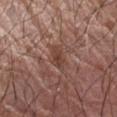A close-up tile cropped from a whole-body skin photograph, about 15 mm across.
The lesion's longest dimension is about 3 mm.
A male patient, in their 70s.
The lesion-visualizer software estimated a footprint of about 3.5 mm², an outline eccentricity of about 0.8 (0 = round, 1 = elongated), and two-axis asymmetry of about 0.3. The software also gave an average lesion color of about L≈41 a*≈21 b*≈24 (CIELAB), about 8 CIELAB-L* units darker than the surrounding skin, and a lesion-to-skin contrast of about 6.5 (normalized; higher = more distinct). It also reported a nevus-likeness score of about 0/100 and lesion-presence confidence of about 90/100.
The lesion is on the left forearm.
This is a white-light tile.